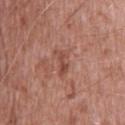notes = catalogued during a skin exam; not biopsied | body site = the upper back | automated lesion analysis = a color-variation rating of about 3/10 and a peripheral color-asymmetry measure near 1; a classifier nevus-likeness of about 0/100 and a lesion-detection confidence of about 100/100 | illumination = white-light illumination | image source = total-body-photography crop, ~15 mm field of view | patient = male, aged around 50 | size = about 3.5 mm.